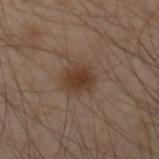Clinical impression: Imaged during a routine full-body skin examination; the lesion was not biopsied and no histopathology is available. Image and clinical context: Captured under cross-polarized illumination. On the left arm. An algorithmic analysis of the crop reported a mean CIELAB color near L≈29 a*≈14 b*≈22, about 7 CIELAB-L* units darker than the surrounding skin, and a normalized lesion–skin contrast near 8.5. The software also gave a classifier nevus-likeness of about 80/100. The patient is a male aged 48 to 52. This image is a 15 mm lesion crop taken from a total-body photograph.